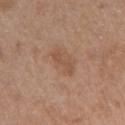{
  "biopsy_status": "not biopsied; imaged during a skin examination",
  "patient": {
    "sex": "female",
    "age_approx": 40
  },
  "lesion_size": {
    "long_diameter_mm_approx": 3.5
  },
  "site": "left upper arm",
  "lighting": "white-light",
  "image": {
    "source": "total-body photography crop",
    "field_of_view_mm": 15
  }
}